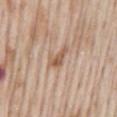<tbp_lesion>
<patient>
  <sex>male</sex>
  <age_approx>65</age_approx>
</patient>
<lighting>white-light</lighting>
<site>mid back</site>
<automated_metrics>
  <area_mm2_approx>3.5</area_mm2_approx>
  <eccentricity>0.9</eccentricity>
  <shape_asymmetry>0.45</shape_asymmetry>
  <nevus_likeness_0_100>0</nevus_likeness_0_100>
  <lesion_detection_confidence_0_100>100</lesion_detection_confidence_0_100>
</automated_metrics>
<lesion_size>
  <long_diameter_mm_approx>3.0</long_diameter_mm_approx>
</lesion_size>
<image>
  <source>total-body photography crop</source>
  <field_of_view_mm>15</field_of_view_mm>
</image>
</tbp_lesion>